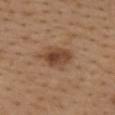workup = imaged on a skin check; not biopsied
image source = ~15 mm crop, total-body skin-cancer survey
automated lesion analysis = a lesion area of about 9 mm² and a shape eccentricity near 0.75; a mean CIELAB color near L≈45 a*≈19 b*≈31 and a normalized lesion–skin contrast near 8; lesion-presence confidence of about 100/100
site = the upper back
lesion diameter = ~4.5 mm (longest diameter)
illumination = white-light
subject = female, approximately 40 years of age